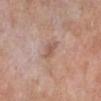  biopsy_status: not biopsied; imaged during a skin examination
  site: left lower leg
  image:
    source: total-body photography crop
    field_of_view_mm: 15
  lesion_size:
    long_diameter_mm_approx: 2.5
  lighting: white-light
  patient:
    sex: male
    age_approx: 80